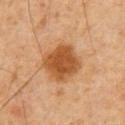<case>
<automated_metrics>
  <cielab_L>43</cielab_L>
  <cielab_a>21</cielab_a>
  <cielab_b>35</cielab_b>
  <vs_skin_darker_L>11.0</vs_skin_darker_L>
  <vs_skin_contrast_norm>9.5</vs_skin_contrast_norm>
  <border_irregularity_0_10>1.5</border_irregularity_0_10>
  <color_variation_0_10>2.5</color_variation_0_10>
  <peripheral_color_asymmetry>1.0</peripheral_color_asymmetry>
  <nevus_likeness_0_100>100</nevus_likeness_0_100>
</automated_metrics>
<patient>
  <sex>male</sex>
  <age_approx>60</age_approx>
</patient>
<lesion_size>
  <long_diameter_mm_approx>4.5</long_diameter_mm_approx>
</lesion_size>
<image>
  <source>total-body photography crop</source>
  <field_of_view_mm>15</field_of_view_mm>
</image>
<site>front of the torso</site>
</case>